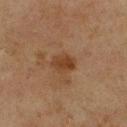illumination: cross-polarized illumination | imaging modality: ~15 mm tile from a whole-body skin photo | patient: male, aged approximately 75 | automated metrics: a lesion color around L≈32 a*≈17 b*≈28 in CIELAB, a lesion–skin lightness drop of about 7, and a lesion-to-skin contrast of about 7.5 (normalized; higher = more distinct); a border-irregularity rating of about 2/10, a within-lesion color-variation index near 3/10, and radial color variation of about 1; a nevus-likeness score of about 40/100 and a lesion-detection confidence of about 100/100 | diameter: ≈3 mm | body site: the front of the torso.